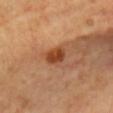- follow-up: imaged on a skin check; not biopsied
- patient: female, roughly 60 years of age
- size: ≈3 mm
- location: the chest
- illumination: cross-polarized illumination
- acquisition: 15 mm crop, total-body photography